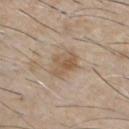The lesion was tiled from a total-body skin photograph and was not biopsied. A male subject about 60 years old. From the chest. The lesion's longest dimension is about 3.5 mm. Imaged with white-light lighting. A lesion tile, about 15 mm wide, cut from a 3D total-body photograph.